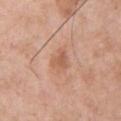Q: Was a biopsy performed?
A: imaged on a skin check; not biopsied
Q: What kind of image is this?
A: total-body-photography crop, ~15 mm field of view
Q: Illumination type?
A: white-light
Q: What are the patient's age and sex?
A: male, in their mid-50s
Q: How large is the lesion?
A: ~2.5 mm (longest diameter)
Q: Lesion location?
A: the chest